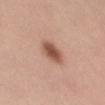Impression: The lesion was photographed on a routine skin check and not biopsied; there is no pathology result. Image and clinical context: This is a white-light tile. The lesion is located on the abdomen. A female patient in their 50s. Cropped from a whole-body photographic skin survey; the tile spans about 15 mm. About 4 mm across. The lesion-visualizer software estimated a mean CIELAB color near L≈53 a*≈22 b*≈29, about 14 CIELAB-L* units darker than the surrounding skin, and a lesion-to-skin contrast of about 9.5 (normalized; higher = more distinct). The analysis additionally found a color-variation rating of about 3/10 and a peripheral color-asymmetry measure near 1. And it measured a nevus-likeness score of about 100/100 and a lesion-detection confidence of about 100/100.